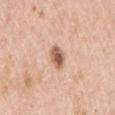<tbp_lesion>
<biopsy_status>not biopsied; imaged during a skin examination</biopsy_status>
</tbp_lesion>